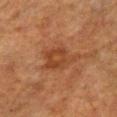Context: About 5 mm across. The patient is a female roughly 55 years of age. From the chest. The tile uses cross-polarized illumination. This image is a 15 mm lesion crop taken from a total-body photograph.